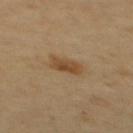This lesion was catalogued during total-body skin photography and was not selected for biopsy. Cropped from a whole-body photographic skin survey; the tile spans about 15 mm. Located on the upper back. The subject is a female aged 58 to 62.